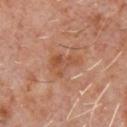This lesion was catalogued during total-body skin photography and was not selected for biopsy.
Longest diameter approximately 4 mm.
The tile uses cross-polarized illumination.
From the chest.
A male patient aged 58–62.
A lesion tile, about 15 mm wide, cut from a 3D total-body photograph.
An algorithmic analysis of the crop reported a border-irregularity index near 5.5/10, internal color variation of about 4.5 on a 0–10 scale, and peripheral color asymmetry of about 1. The analysis additionally found a nevus-likeness score of about 0/100 and a lesion-detection confidence of about 100/100.